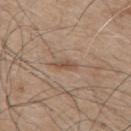follow-up = total-body-photography surveillance lesion; no biopsy
subject = male, in their mid-70s
acquisition = total-body-photography crop, ~15 mm field of view
illumination = white-light illumination
body site = the mid back